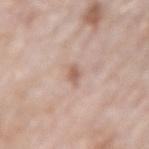follow-up=no biopsy performed (imaged during a skin exam)
lesion size=~2.5 mm (longest diameter)
lighting=white-light illumination
patient=male, approximately 75 years of age
acquisition=15 mm crop, total-body photography
anatomic site=the arm